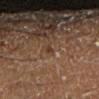Assessment: This lesion was catalogued during total-body skin photography and was not selected for biopsy. Background: The lesion's longest dimension is about 1 mm. The total-body-photography lesion software estimated an average lesion color of about L≈32 a*≈18 b*≈26 (CIELAB) and about 7 CIELAB-L* units darker than the surrounding skin. The analysis additionally found a border-irregularity index near 3.5/10, a color-variation rating of about 0/10, and a peripheral color-asymmetry measure near 0. The analysis additionally found a classifier nevus-likeness of about 0/100 and lesion-presence confidence of about 95/100. Captured under cross-polarized illumination. The subject is a male aged around 60. The lesion is located on the right lower leg. Cropped from a total-body skin-imaging series; the visible field is about 15 mm.